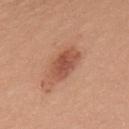No biopsy was performed on this lesion — it was imaged during a full skin examination and was not determined to be concerning. Captured under white-light illumination. The subject is a female aged around 35. The lesion is on the upper back. The lesion-visualizer software estimated a footprint of about 9.5 mm², an eccentricity of roughly 0.8, and a shape-asymmetry score of about 0.15 (0 = symmetric). The software also gave a lesion color around L≈53 a*≈25 b*≈31 in CIELAB and about 11 CIELAB-L* units darker than the surrounding skin. The analysis additionally found a nevus-likeness score of about 95/100 and lesion-presence confidence of about 100/100. A 15 mm crop from a total-body photograph taken for skin-cancer surveillance. About 4.5 mm across.